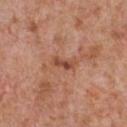Longest diameter approximately 3 mm. The lesion is located on the chest. The subject is a male aged 63–67. Imaged with white-light lighting. A 15 mm crop from a total-body photograph taken for skin-cancer surveillance.